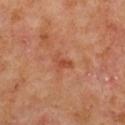Impression: Imaged during a routine full-body skin examination; the lesion was not biopsied and no histopathology is available. Image and clinical context: A female patient, roughly 50 years of age. This is a cross-polarized tile. The lesion is on the chest. A lesion tile, about 15 mm wide, cut from a 3D total-body photograph.